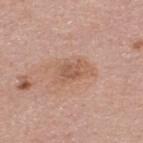This lesion was catalogued during total-body skin photography and was not selected for biopsy. Automated image analysis of the tile measured a footprint of about 8.5 mm², an outline eccentricity of about 0.8 (0 = round, 1 = elongated), and a symmetry-axis asymmetry near 0.25. The software also gave a mean CIELAB color near L≈57 a*≈21 b*≈30 and about 8 CIELAB-L* units darker than the surrounding skin. And it measured border irregularity of about 3 on a 0–10 scale, a color-variation rating of about 2.5/10, and radial color variation of about 1. A male subject aged approximately 45. A lesion tile, about 15 mm wide, cut from a 3D total-body photograph. On the upper back. This is a white-light tile. Measured at roughly 4.5 mm in maximum diameter.